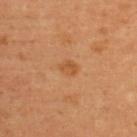{
  "biopsy_status": "not biopsied; imaged during a skin examination",
  "patient": {
    "sex": "female",
    "age_approx": 35
  },
  "lighting": "cross-polarized",
  "image": {
    "source": "total-body photography crop",
    "field_of_view_mm": 15
  },
  "site": "upper back"
}